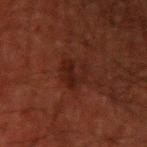Clinical impression: Captured during whole-body skin photography for melanoma surveillance; the lesion was not biopsied. Acquisition and patient details: Imaged with cross-polarized lighting. The patient is a male in their 50s. A 15 mm close-up tile from a total-body photography series done for melanoma screening. From the left upper arm.